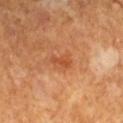Findings:
• workup — catalogued during a skin exam; not biopsied
• body site — the left lower leg
• subject — male, aged 58–62
• imaging modality — 15 mm crop, total-body photography